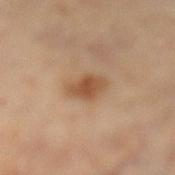subject: female, aged 58–62
location: the left lower leg
image-analysis metrics: a shape eccentricity near 0.7; an average lesion color of about L≈51 a*≈20 b*≈34 (CIELAB) and roughly 11 lightness units darker than nearby skin; a border-irregularity index near 2/10, a within-lesion color-variation index near 3/10, and radial color variation of about 1; an automated nevus-likeness rating near 80 out of 100 and a lesion-detection confidence of about 100/100
lesion diameter: ≈3 mm
illumination: cross-polarized illumination
imaging modality: 15 mm crop, total-body photography